A female patient, roughly 60 years of age · from the upper back · cropped from a total-body skin-imaging series; the visible field is about 15 mm: 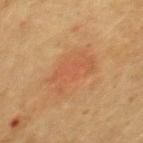* biopsy diagnosis · a nodular basal cell carcinoma (malignant)